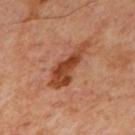<record>
  <biopsy_status>not biopsied; imaged during a skin examination</biopsy_status>
  <lesion_size>
    <long_diameter_mm_approx>6.5</long_diameter_mm_approx>
  </lesion_size>
  <automated_metrics>
    <eccentricity>0.95</eccentricity>
    <shape_asymmetry>0.45</shape_asymmetry>
  </automated_metrics>
  <site>mid back</site>
  <image>
    <source>total-body photography crop</source>
    <field_of_view_mm>15</field_of_view_mm>
  </image>
  <patient>
    <age_approx>65</age_approx>
  </patient>
  <lighting>cross-polarized</lighting>
</record>